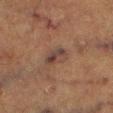Impression:
This lesion was catalogued during total-body skin photography and was not selected for biopsy.
Clinical summary:
Longest diameter approximately 3 mm. The tile uses cross-polarized illumination. A male patient about 75 years old. From the right thigh. Cropped from a whole-body photographic skin survey; the tile spans about 15 mm.